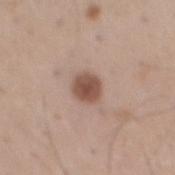Part of a total-body skin-imaging series; this lesion was reviewed on a skin check and was not flagged for biopsy. This image is a 15 mm lesion crop taken from a total-body photograph. On the back. The lesion's longest dimension is about 3 mm. Automated image analysis of the tile measured a nevus-likeness score of about 95/100. The tile uses white-light illumination. A male patient aged 48 to 52.